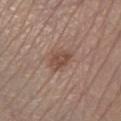Case summary:
• notes: imaged on a skin check; not biopsied
• body site: the left lower leg
• illumination: white-light
• image source: total-body-photography crop, ~15 mm field of view
• subject: male, aged approximately 65